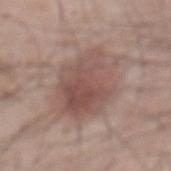Assessment:
Recorded during total-body skin imaging; not selected for excision or biopsy.
Image and clinical context:
The lesion is located on the abdomen. The total-body-photography lesion software estimated an automated nevus-likeness rating near 85 out of 100. Imaged with white-light lighting. A male subject aged approximately 60. A 15 mm crop from a total-body photograph taken for skin-cancer surveillance.